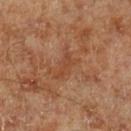Q: Was this lesion biopsied?
A: no biopsy performed (imaged during a skin exam)
Q: Who is the patient?
A: male, aged 68–72
Q: How large is the lesion?
A: about 3 mm
Q: What is the imaging modality?
A: total-body-photography crop, ~15 mm field of view
Q: What lighting was used for the tile?
A: cross-polarized
Q: What is the anatomic site?
A: the left lower leg